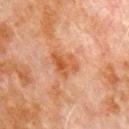Part of a total-body skin-imaging series; this lesion was reviewed on a skin check and was not flagged for biopsy.
On the chest.
Approximately 4 mm at its widest.
The patient is a male approximately 80 years of age.
Imaged with cross-polarized lighting.
A 15 mm close-up tile from a total-body photography series done for melanoma screening.
Automated tile analysis of the lesion measured roughly 8 lightness units darker than nearby skin and a normalized lesion–skin contrast near 8. And it measured a detector confidence of about 100 out of 100 that the crop contains a lesion.